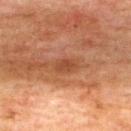<record>
<biopsy_status>not biopsied; imaged during a skin examination</biopsy_status>
<site>upper back</site>
<lesion_size>
  <long_diameter_mm_approx>3.0</long_diameter_mm_approx>
</lesion_size>
<lighting>cross-polarized</lighting>
<image>
  <source>total-body photography crop</source>
  <field_of_view_mm>15</field_of_view_mm>
</image>
<patient>
  <sex>male</sex>
  <age_approx>75</age_approx>
</patient>
</record>